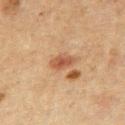A male subject aged 48–52. From the chest. A region of skin cropped from a whole-body photographic capture, roughly 15 mm wide.